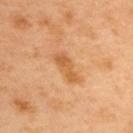The lesion was tiled from a total-body skin photograph and was not biopsied. A male subject aged around 50. The lesion is on the upper back. A 15 mm close-up tile from a total-body photography series done for melanoma screening.